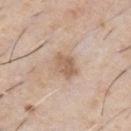Clinical impression: No biopsy was performed on this lesion — it was imaged during a full skin examination and was not determined to be concerning. Acquisition and patient details: Cropped from a whole-body photographic skin survey; the tile spans about 15 mm. A male patient, aged 58–62. Measured at roughly 3 mm in maximum diameter. This is a white-light tile. The lesion is on the chest.